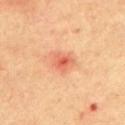patient:
  sex: male
  age_approx: 70
lesion_size:
  long_diameter_mm_approx: 2.5
image:
  source: total-body photography crop
  field_of_view_mm: 15
lighting: cross-polarized
site: chest